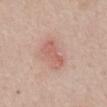<record>
  <biopsy_status>not biopsied; imaged during a skin examination</biopsy_status>
  <site>abdomen</site>
  <image>
    <source>total-body photography crop</source>
    <field_of_view_mm>15</field_of_view_mm>
  </image>
  <patient>
    <sex>male</sex>
    <age_approx>70</age_approx>
  </patient>
</record>